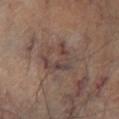| field | value |
|---|---|
| workup | no biopsy performed (imaged during a skin exam) |
| lesion diameter | ≈4 mm |
| site | the leg |
| subject | male, aged approximately 65 |
| illumination | cross-polarized |
| image | ~15 mm crop, total-body skin-cancer survey |